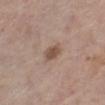Part of a total-body skin-imaging series; this lesion was reviewed on a skin check and was not flagged for biopsy.
Imaged with white-light lighting.
The lesion's longest dimension is about 3 mm.
A female subject approximately 55 years of age.
Located on the left lower leg.
This image is a 15 mm lesion crop taken from a total-body photograph.
The lesion-visualizer software estimated a mean CIELAB color near L≈51 a*≈17 b*≈26, about 10 CIELAB-L* units darker than the surrounding skin, and a lesion-to-skin contrast of about 7.5 (normalized; higher = more distinct). The analysis additionally found a border-irregularity index near 2/10, internal color variation of about 2.5 on a 0–10 scale, and peripheral color asymmetry of about 1.